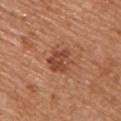Clinical summary: A male subject aged around 70. From the upper back. A 15 mm close-up extracted from a 3D total-body photography capture. Imaged with white-light lighting.